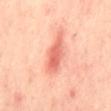Impression:
Recorded during total-body skin imaging; not selected for excision or biopsy.
Clinical summary:
Captured under cross-polarized illumination. From the mid back. A region of skin cropped from a whole-body photographic capture, roughly 15 mm wide. Automated tile analysis of the lesion measured a border-irregularity rating of about 3.5/10, a color-variation rating of about 3.5/10, and a peripheral color-asymmetry measure near 1. The software also gave an automated nevus-likeness rating near 55 out of 100 and lesion-presence confidence of about 100/100. A female subject, aged approximately 40.